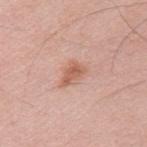Assessment:
This lesion was catalogued during total-body skin photography and was not selected for biopsy.